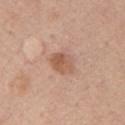| field | value |
|---|---|
| workup | catalogued during a skin exam; not biopsied |